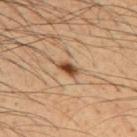| key | value |
|---|---|
| follow-up | imaged on a skin check; not biopsied |
| automated lesion analysis | a footprint of about 3.5 mm², an eccentricity of roughly 0.85, and two-axis asymmetry of about 0.2; a nevus-likeness score of about 95/100 and a lesion-detection confidence of about 100/100 |
| image | total-body-photography crop, ~15 mm field of view |
| location | the mid back |
| tile lighting | cross-polarized illumination |
| size | ~2.5 mm (longest diameter) |
| patient | male, aged around 50 |